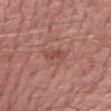Part of a total-body skin-imaging series; this lesion was reviewed on a skin check and was not flagged for biopsy. A male patient, roughly 60 years of age. The tile uses white-light illumination. The lesion is located on the arm. The lesion's longest dimension is about 3 mm. Cropped from a whole-body photographic skin survey; the tile spans about 15 mm.